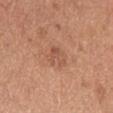{
  "biopsy_status": "not biopsied; imaged during a skin examination",
  "image": {
    "source": "total-body photography crop",
    "field_of_view_mm": 15
  },
  "lesion_size": {
    "long_diameter_mm_approx": 2.5
  },
  "lighting": "white-light",
  "patient": {
    "sex": "male",
    "age_approx": 30
  },
  "site": "chest"
}